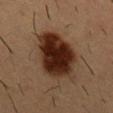Captured during whole-body skin photography for melanoma surveillance; the lesion was not biopsied. From the chest. A male subject, about 55 years old. Cropped from a total-body skin-imaging series; the visible field is about 15 mm. This is a cross-polarized tile.